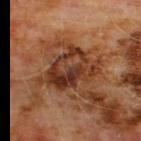workup: total-body-photography surveillance lesion; no biopsy
image source: 15 mm crop, total-body photography
site: the chest
patient: male, aged around 60
lighting: cross-polarized illumination